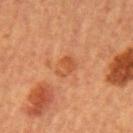Clinical impression: Captured during whole-body skin photography for melanoma surveillance; the lesion was not biopsied. Context: About 3 mm across. A female patient in their mid-50s. A roughly 15 mm field-of-view crop from a total-body skin photograph. The tile uses cross-polarized illumination. From the left upper arm.